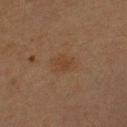The lesion is on the arm. Captured under cross-polarized illumination. A roughly 15 mm field-of-view crop from a total-body skin photograph. The recorded lesion diameter is about 3.5 mm. A female patient aged around 55. The lesion-visualizer software estimated an area of roughly 6.5 mm². It also reported an average lesion color of about L≈33 a*≈14 b*≈26 (CIELAB) and a normalized border contrast of about 5. It also reported a lesion-detection confidence of about 100/100.